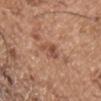Q: Was this lesion biopsied?
A: imaged on a skin check; not biopsied